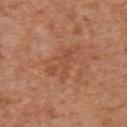Impression:
Part of a total-body skin-imaging series; this lesion was reviewed on a skin check and was not flagged for biopsy.
Acquisition and patient details:
Cropped from a whole-body photographic skin survey; the tile spans about 15 mm. From the upper back. A male subject approximately 65 years of age.